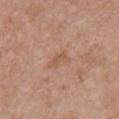{"biopsy_status": "not biopsied; imaged during a skin examination", "site": "chest", "lesion_size": {"long_diameter_mm_approx": 3.0}, "automated_metrics": {"area_mm2_approx": 3.5, "shape_asymmetry": 0.35, "border_irregularity_0_10": 4.0, "color_variation_0_10": 0.5, "lesion_detection_confidence_0_100": 100}, "image": {"source": "total-body photography crop", "field_of_view_mm": 15}, "patient": {"sex": "female", "age_approx": 40}}